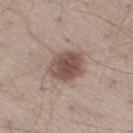Case summary:
• notes: no biopsy performed (imaged during a skin exam)
• tile lighting: white-light illumination
• size: ~4 mm (longest diameter)
• acquisition: total-body-photography crop, ~15 mm field of view
• anatomic site: the right thigh
• subject: male, aged 28–32